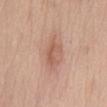biopsy_status: not biopsied; imaged during a skin examination